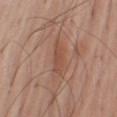Case summary:
* patient — male, aged around 70
* image — ~15 mm crop, total-body skin-cancer survey
* anatomic site — the right upper arm